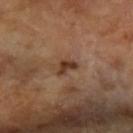The lesion was tiled from a total-body skin photograph and was not biopsied.
A 15 mm crop from a total-body photograph taken for skin-cancer surveillance.
A female subject aged around 70.
The lesion is located on the arm.
The recorded lesion diameter is about 3 mm.